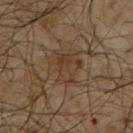Captured during whole-body skin photography for melanoma surveillance; the lesion was not biopsied. The subject is a male aged 63 to 67. The tile uses cross-polarized illumination. A 15 mm close-up tile from a total-body photography series done for melanoma screening. The lesion is on the upper back. The lesion's longest dimension is about 4 mm.